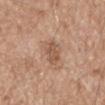{"biopsy_status": "not biopsied; imaged during a skin examination", "image": {"source": "total-body photography crop", "field_of_view_mm": 15}, "site": "mid back", "lesion_size": {"long_diameter_mm_approx": 4.0}, "patient": {"sex": "male", "age_approx": 75}, "lighting": "white-light"}